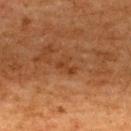The lesion was tiled from a total-body skin photograph and was not biopsied.
Imaged with cross-polarized lighting.
The subject is a female about 50 years old.
The lesion is located on the upper back.
A close-up tile cropped from a whole-body skin photograph, about 15 mm across.